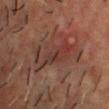workup: total-body-photography surveillance lesion; no biopsy | image-analysis metrics: a lesion area of about 11 mm², an outline eccentricity of about 0.9 (0 = round, 1 = elongated), and a symmetry-axis asymmetry near 0.4; a lesion color around L≈29 a*≈19 b*≈20 in CIELAB and a normalized border contrast of about 6.5; a border-irregularity rating of about 7/10, a within-lesion color-variation index near 5.5/10, and peripheral color asymmetry of about 1.5; an automated nevus-likeness rating near 0 out of 100 and lesion-presence confidence of about 50/100 | site: the chest | tile lighting: cross-polarized illumination | diameter: about 6 mm | image: ~15 mm crop, total-body skin-cancer survey | subject: male, about 60 years old.